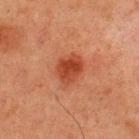workup: catalogued during a skin exam; not biopsied
automated lesion analysis: a border-irregularity rating of about 2/10, internal color variation of about 3.5 on a 0–10 scale, and peripheral color asymmetry of about 1
patient: male, aged 58 to 62
anatomic site: the upper back
image source: ~15 mm tile from a whole-body skin photo
diameter: ~3.5 mm (longest diameter)